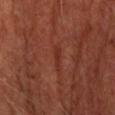workup=no biopsy performed (imaged during a skin exam)
lighting=cross-polarized illumination
patient=male, aged 63–67
site=the head or neck
image=15 mm crop, total-body photography
lesion size=about 3 mm